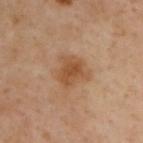workup = no biopsy performed (imaged during a skin exam) | subject = male, about 55 years old | body site = the upper back | TBP lesion metrics = a border-irregularity index near 3.5/10 and a peripheral color-asymmetry measure near 1 | image source = ~15 mm crop, total-body skin-cancer survey | tile lighting = cross-polarized | diameter = about 4 mm.